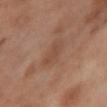<lesion>
  <biopsy_status>not biopsied; imaged during a skin examination</biopsy_status>
  <site>right thigh</site>
  <patient>
    <sex>female</sex>
    <age_approx>55</age_approx>
  </patient>
  <image>
    <source>total-body photography crop</source>
    <field_of_view_mm>15</field_of_view_mm>
  </image>
  <automated_metrics>
    <border_irregularity_0_10>2.5</border_irregularity_0_10>
    <color_variation_0_10>1.5</color_variation_0_10>
    <peripheral_color_asymmetry>0.5</peripheral_color_asymmetry>
  </automated_metrics>
</lesion>